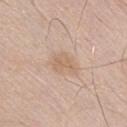biopsy_status: not biopsied; imaged during a skin examination
site: front of the torso
patient:
  sex: male
  age_approx: 30
automated_metrics:
  area_mm2_approx: 5.0
  eccentricity: 0.7
  shape_asymmetry: 0.4
  vs_skin_darker_L: 7.0
  vs_skin_contrast_norm: 5.5
  border_irregularity_0_10: 4.0
  color_variation_0_10: 2.0
  peripheral_color_asymmetry: 1.0
lesion_size:
  long_diameter_mm_approx: 3.0
image:
  source: total-body photography crop
  field_of_view_mm: 15
lighting: white-light